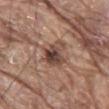Q: Was a biopsy performed?
A: total-body-photography surveillance lesion; no biopsy
Q: Lesion size?
A: ≈3.5 mm
Q: Illumination type?
A: white-light
Q: Lesion location?
A: the mid back
Q: How was this image acquired?
A: ~15 mm crop, total-body skin-cancer survey
Q: Automated lesion metrics?
A: a lesion area of about 8.5 mm² and an outline eccentricity of about 0.35 (0 = round, 1 = elongated); a lesion color around L≈44 a*≈18 b*≈25 in CIELAB, roughly 12 lightness units darker than nearby skin, and a lesion-to-skin contrast of about 9.5 (normalized; higher = more distinct); a within-lesion color-variation index near 8.5/10 and peripheral color asymmetry of about 3.5; an automated nevus-likeness rating near 0 out of 100 and lesion-presence confidence of about 100/100
Q: Who is the patient?
A: male, aged 78–82